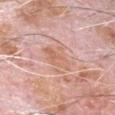This lesion was catalogued during total-body skin photography and was not selected for biopsy.
From the head or neck.
The subject is a male roughly 80 years of age.
A roughly 15 mm field-of-view crop from a total-body skin photograph.